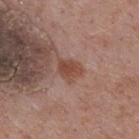Assessment: The lesion was photographed on a routine skin check and not biopsied; there is no pathology result. Background: A roughly 15 mm field-of-view crop from a total-body skin photograph. From the upper back. Captured under white-light illumination. A male patient roughly 70 years of age. Longest diameter approximately 3 mm. The lesion-visualizer software estimated a lesion color around L≈47 a*≈22 b*≈27 in CIELAB, a lesion–skin lightness drop of about 9, and a normalized lesion–skin contrast near 8. It also reported a border-irregularity rating of about 2.5/10 and a color-variation rating of about 2.5/10.